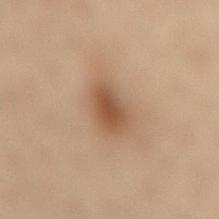Recorded during total-body skin imaging; not selected for excision or biopsy. Imaged with cross-polarized lighting. Cropped from a whole-body photographic skin survey; the tile spans about 15 mm. A female patient, in their mid- to late 50s. Measured at roughly 3.5 mm in maximum diameter. The lesion is on the lower back.